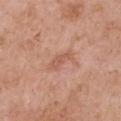| key | value |
|---|---|
| biopsy status | catalogued during a skin exam; not biopsied |
| lesion diameter | about 3 mm |
| subject | female, in their 60s |
| image | 15 mm crop, total-body photography |
| image-analysis metrics | a mean CIELAB color near L≈57 a*≈24 b*≈30 and a normalized border contrast of about 5 |
| illumination | white-light |
| location | the chest |